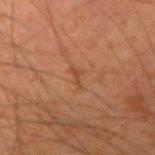– biopsy status: imaged on a skin check; not biopsied
– patient: male, approximately 35 years of age
– acquisition: ~15 mm crop, total-body skin-cancer survey
– size: about 2.5 mm
– location: the right upper arm